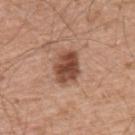Clinical impression:
No biopsy was performed on this lesion — it was imaged during a full skin examination and was not determined to be concerning.
Context:
A male patient in their mid- to late 50s. A 15 mm close-up tile from a total-body photography series done for melanoma screening. The lesion is located on the back. The lesion's longest dimension is about 3.5 mm. Automated tile analysis of the lesion measured a classifier nevus-likeness of about 80/100 and a detector confidence of about 100 out of 100 that the crop contains a lesion.